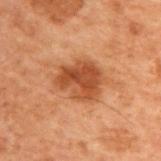The lesion was tiled from a total-body skin photograph and was not biopsied.
The total-body-photography lesion software estimated an outline eccentricity of about 0.55 (0 = round, 1 = elongated). The software also gave a lesion color around L≈38 a*≈22 b*≈31 in CIELAB, about 10 CIELAB-L* units darker than the surrounding skin, and a normalized border contrast of about 8.5.
A male patient, aged approximately 70.
The lesion's longest dimension is about 4.5 mm.
The lesion is on the upper back.
This is a cross-polarized tile.
A 15 mm close-up extracted from a 3D total-body photography capture.